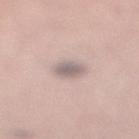Part of a total-body skin-imaging series; this lesion was reviewed on a skin check and was not flagged for biopsy. About 3 mm across. A female subject, aged 28–32. From the right lower leg. A 15 mm close-up tile from a total-body photography series done for melanoma screening. Captured under white-light illumination.